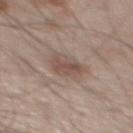The lesion was tiled from a total-body skin photograph and was not biopsied.
A 15 mm close-up tile from a total-body photography series done for melanoma screening.
Measured at roughly 4 mm in maximum diameter.
The lesion-visualizer software estimated a classifier nevus-likeness of about 40/100.
The lesion is located on the mid back.
A male patient aged around 50.
This is a white-light tile.